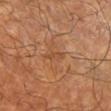Impression:
The lesion was tiled from a total-body skin photograph and was not biopsied.
Image and clinical context:
A male patient, about 60 years old. The lesion is located on the right lower leg. The lesion's longest dimension is about 3 mm. The lesion-visualizer software estimated a footprint of about 2.5 mm² and a shape-asymmetry score of about 0.45 (0 = symmetric). A 15 mm close-up tile from a total-body photography series done for melanoma screening.